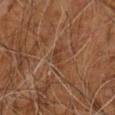Clinical impression:
This lesion was catalogued during total-body skin photography and was not selected for biopsy.
Image and clinical context:
The recorded lesion diameter is about 3 mm. Captured under cross-polarized illumination. Cropped from a whole-body photographic skin survey; the tile spans about 15 mm. The subject is a male aged approximately 60. The lesion-visualizer software estimated an area of roughly 3 mm², an eccentricity of roughly 0.9, and two-axis asymmetry of about 0.35. It also reported a lesion color around L≈37 a*≈21 b*≈31 in CIELAB and a normalized lesion–skin contrast near 5.5. On the right arm.